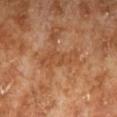Impression:
Captured during whole-body skin photography for melanoma surveillance; the lesion was not biopsied.
Context:
The lesion is on the left lower leg. A 15 mm close-up tile from a total-body photography series done for melanoma screening. A male subject aged around 70.